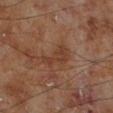Imaged during a routine full-body skin examination; the lesion was not biopsied and no histopathology is available.
The lesion is on the left lower leg.
A 15 mm close-up extracted from a 3D total-body photography capture.
The tile uses cross-polarized illumination.
Measured at roughly 4 mm in maximum diameter.
A male patient aged 68–72.